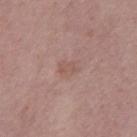Case summary:
• workup — total-body-photography surveillance lesion; no biopsy
• image — 15 mm crop, total-body photography
• patient — male, aged around 65
• size — about 2.5 mm
• lighting — white-light illumination
• anatomic site — the chest